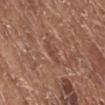Q: Was a biopsy performed?
A: no biopsy performed (imaged during a skin exam)
Q: Where on the body is the lesion?
A: the right lower leg
Q: What is the imaging modality?
A: ~15 mm tile from a whole-body skin photo
Q: What are the patient's age and sex?
A: female, in their mid-70s
Q: Lesion size?
A: about 2.5 mm
Q: Illumination type?
A: white-light illumination
Q: Automated lesion metrics?
A: border irregularity of about 4.5 on a 0–10 scale, internal color variation of about 0 on a 0–10 scale, and a peripheral color-asymmetry measure near 0